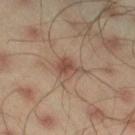| key | value |
|---|---|
| notes | total-body-photography surveillance lesion; no biopsy |
| image | ~15 mm crop, total-body skin-cancer survey |
| site | the leg |
| diameter | ~3 mm (longest diameter) |
| TBP lesion metrics | a mean CIELAB color near L≈48 a*≈18 b*≈26, about 9 CIELAB-L* units darker than the surrounding skin, and a normalized border contrast of about 7; a classifier nevus-likeness of about 50/100 and lesion-presence confidence of about 100/100 |
| subject | male, aged around 45 |